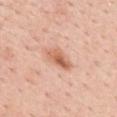Q: Is there a histopathology result?
A: catalogued during a skin exam; not biopsied
Q: What did automated image analysis measure?
A: a lesion color around L≈63 a*≈25 b*≈33 in CIELAB, roughly 12 lightness units darker than nearby skin, and a lesion-to-skin contrast of about 7.5 (normalized; higher = more distinct); a nevus-likeness score of about 75/100
Q: What are the patient's age and sex?
A: female, approximately 45 years of age
Q: What is the anatomic site?
A: the upper back
Q: What lighting was used for the tile?
A: white-light
Q: What kind of image is this?
A: ~15 mm tile from a whole-body skin photo
Q: What is the lesion's diameter?
A: ≈4 mm